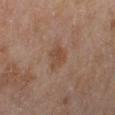No biopsy was performed on this lesion — it was imaged during a full skin examination and was not determined to be concerning. This image is a 15 mm lesion crop taken from a total-body photograph. The lesion-visualizer software estimated roughly 6 lightness units darker than nearby skin and a lesion-to-skin contrast of about 6 (normalized; higher = more distinct). The software also gave a border-irregularity rating of about 3/10. The patient is a female aged approximately 60. The lesion is on the left lower leg. Captured under cross-polarized illumination. The lesion's longest dimension is about 2.5 mm.